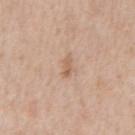biopsy status=catalogued during a skin exam; not biopsied | patient=male, about 65 years old | anatomic site=the chest | lesion diameter=about 2.5 mm | tile lighting=white-light illumination | image=15 mm crop, total-body photography.